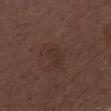Recorded during total-body skin imaging; not selected for excision or biopsy. The tile uses white-light illumination. The lesion is located on the right forearm. Longest diameter approximately 2.5 mm. A female subject approximately 50 years of age. A 15 mm close-up extracted from a 3D total-body photography capture. Automated image analysis of the tile measured a lesion area of about 3 mm², a shape eccentricity near 0.85, and a shape-asymmetry score of about 0.4 (0 = symmetric). And it measured a mean CIELAB color near L≈30 a*≈17 b*≈22 and a normalized lesion–skin contrast near 4.5. The analysis additionally found a border-irregularity rating of about 4/10, internal color variation of about 0.5 on a 0–10 scale, and a peripheral color-asymmetry measure near 0. And it measured lesion-presence confidence of about 100/100.